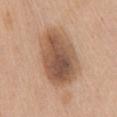follow-up = catalogued during a skin exam; not biopsied | lesion size = about 8 mm | anatomic site = the chest | image source = total-body-photography crop, ~15 mm field of view | patient = female, approximately 65 years of age | image-analysis metrics = border irregularity of about 2 on a 0–10 scale, a color-variation rating of about 7/10, and a peripheral color-asymmetry measure near 2.5; a nevus-likeness score of about 50/100 and lesion-presence confidence of about 100/100 | lighting = white-light illumination.